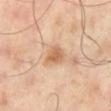This lesion was catalogued during total-body skin photography and was not selected for biopsy. Captured under cross-polarized illumination. The patient is a male aged 63–67. Approximately 3 mm at its widest. The lesion-visualizer software estimated a nevus-likeness score of about 40/100 and a lesion-detection confidence of about 100/100. From the right lower leg. This image is a 15 mm lesion crop taken from a total-body photograph.